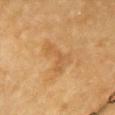Captured during whole-body skin photography for melanoma surveillance; the lesion was not biopsied.
From the chest.
A female subject aged approximately 55.
This is a cross-polarized tile.
Longest diameter approximately 5 mm.
A close-up tile cropped from a whole-body skin photograph, about 15 mm across.